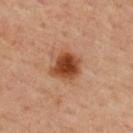Recorded during total-body skin imaging; not selected for excision or biopsy. The lesion is on the back. A 15 mm close-up extracted from a 3D total-body photography capture. A male subject, aged 53 to 57.